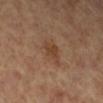| feature | finding |
|---|---|
| location | the right lower leg |
| subject | female, aged 68–72 |
| TBP lesion metrics | an area of roughly 6 mm² and an outline eccentricity of about 0.8 (0 = round, 1 = elongated); a lesion color around L≈43 a*≈19 b*≈31 in CIELAB, a lesion–skin lightness drop of about 7, and a lesion-to-skin contrast of about 6 (normalized; higher = more distinct); a nevus-likeness score of about 10/100 and lesion-presence confidence of about 100/100 |
| size | ~3.5 mm (longest diameter) |
| acquisition | ~15 mm tile from a whole-body skin photo |
| illumination | cross-polarized |